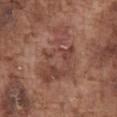The lesion was photographed on a routine skin check and not biopsied; there is no pathology result.
On the front of the torso.
Automated tile analysis of the lesion measured a footprint of about 24 mm², a shape eccentricity near 0.8, and two-axis asymmetry of about 0.45. The analysis additionally found a lesion color around L≈45 a*≈22 b*≈25 in CIELAB, roughly 7 lightness units darker than nearby skin, and a lesion-to-skin contrast of about 5.5 (normalized; higher = more distinct).
This is a white-light tile.
A region of skin cropped from a whole-body photographic capture, roughly 15 mm wide.
A male patient aged around 75.